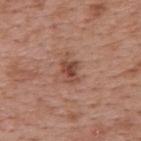Notes:
* workup: catalogued during a skin exam; not biopsied
* illumination: white-light
* image: ~15 mm tile from a whole-body skin photo
* subject: female, aged 38 to 42
* anatomic site: the upper back
* size: ≈3.5 mm
* automated metrics: a lesion area of about 5.5 mm², an eccentricity of roughly 0.8, and a symmetry-axis asymmetry near 0.35; an average lesion color of about L≈47 a*≈23 b*≈28 (CIELAB), roughly 10 lightness units darker than nearby skin, and a lesion-to-skin contrast of about 7.5 (normalized; higher = more distinct)